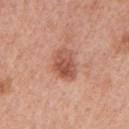Context:
A female patient aged 58 to 62. A 15 mm crop from a total-body photograph taken for skin-cancer surveillance. The tile uses white-light illumination. Measured at roughly 3.5 mm in maximum diameter. The lesion is on the arm.